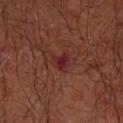Imaged during a routine full-body skin examination; the lesion was not biopsied and no histopathology is available.
The lesion is on the left forearm.
Imaged with cross-polarized lighting.
A male patient, aged approximately 65.
A roughly 15 mm field-of-view crop from a total-body skin photograph.
Longest diameter approximately 2.5 mm.